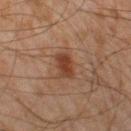Impression: Recorded during total-body skin imaging; not selected for excision or biopsy. Context: Automated tile analysis of the lesion measured a lesion area of about 5 mm² and a shape-asymmetry score of about 0.3 (0 = symmetric). The software also gave a mean CIELAB color near L≈32 a*≈19 b*≈26, about 9 CIELAB-L* units darker than the surrounding skin, and a normalized border contrast of about 8.5. It also reported internal color variation of about 2 on a 0–10 scale and radial color variation of about 0.5. The software also gave an automated nevus-likeness rating near 85 out of 100. Captured under cross-polarized illumination. The lesion is located on the left thigh. The patient is a male aged around 45. A lesion tile, about 15 mm wide, cut from a 3D total-body photograph. Longest diameter approximately 3 mm.